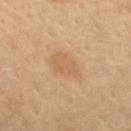{
  "biopsy_status": "not biopsied; imaged during a skin examination",
  "site": "mid back",
  "image": {
    "source": "total-body photography crop",
    "field_of_view_mm": 15
  },
  "patient": {
    "age_approx": 65
  }
}